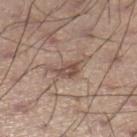The lesion was photographed on a routine skin check and not biopsied; there is no pathology result. From the leg. The lesion's longest dimension is about 4.5 mm. A close-up tile cropped from a whole-body skin photograph, about 15 mm across. An algorithmic analysis of the crop reported an automated nevus-likeness rating near 0 out of 100 and a detector confidence of about 60 out of 100 that the crop contains a lesion. A male subject aged 53 to 57. The tile uses white-light illumination.